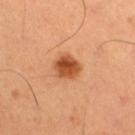This lesion was catalogued during total-body skin photography and was not selected for biopsy.
A male patient roughly 50 years of age.
The total-body-photography lesion software estimated a lesion area of about 7 mm², a shape eccentricity near 0.55, and a symmetry-axis asymmetry near 0.2. And it measured a mean CIELAB color near L≈51 a*≈29 b*≈40 and roughly 15 lightness units darker than nearby skin. And it measured peripheral color asymmetry of about 2.
Imaged with cross-polarized lighting.
A roughly 15 mm field-of-view crop from a total-body skin photograph.
The lesion's longest dimension is about 3.5 mm.
Located on the right thigh.